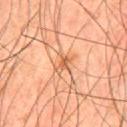Recorded during total-body skin imaging; not selected for excision or biopsy.
Measured at roughly 2.5 mm in maximum diameter.
Imaged with cross-polarized lighting.
Cropped from a whole-body photographic skin survey; the tile spans about 15 mm.
An algorithmic analysis of the crop reported an area of roughly 3 mm². It also reported border irregularity of about 5 on a 0–10 scale, a within-lesion color-variation index near 1/10, and a peripheral color-asymmetry measure near 0.
The subject is a male in their mid- to late 40s.
The lesion is on the mid back.